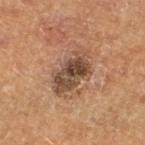illumination: cross-polarized; imaging modality: 15 mm crop, total-body photography; subject: male, approximately 65 years of age; site: the left lower leg.